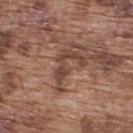Captured during whole-body skin photography for melanoma surveillance; the lesion was not biopsied. Measured at roughly 5 mm in maximum diameter. From the upper back. A 15 mm crop from a total-body photograph taken for skin-cancer surveillance. A male subject in their mid- to late 70s. Automated image analysis of the tile measured a border-irregularity index near 10/10, a color-variation rating of about 1/10, and peripheral color asymmetry of about 0. The software also gave a nevus-likeness score of about 0/100 and a lesion-detection confidence of about 60/100.